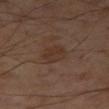workup: no biopsy performed (imaged during a skin exam)
subject: male, aged 53–57
imaging modality: total-body-photography crop, ~15 mm field of view
tile lighting: cross-polarized illumination
TBP lesion metrics: border irregularity of about 1.5 on a 0–10 scale and internal color variation of about 2 on a 0–10 scale
size: ≈3 mm
body site: the right thigh